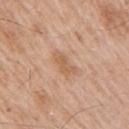patient:
  sex: male
  age_approx: 60
lesion_size:
  long_diameter_mm_approx: 3.5
site: right upper arm
image:
  source: total-body photography crop
  field_of_view_mm: 15
lighting: white-light